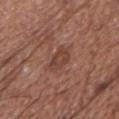Clinical impression:
Imaged during a routine full-body skin examination; the lesion was not biopsied and no histopathology is available.